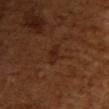<lesion>
  <biopsy_status>not biopsied; imaged during a skin examination</biopsy_status>
  <automated_metrics>
    <cielab_L>24</cielab_L>
    <cielab_a>20</cielab_a>
    <cielab_b>28</cielab_b>
    <vs_skin_darker_L>5.0</vs_skin_darker_L>
    <vs_skin_contrast_norm>6.0</vs_skin_contrast_norm>
    <color_variation_0_10>1.5</color_variation_0_10>
    <peripheral_color_asymmetry>0.5</peripheral_color_asymmetry>
  </automated_metrics>
  <site>upper back</site>
  <lesion_size>
    <long_diameter_mm_approx>2.5</long_diameter_mm_approx>
  </lesion_size>
  <image>
    <source>total-body photography crop</source>
    <field_of_view_mm>15</field_of_view_mm>
  </image>
  <lighting>cross-polarized</lighting>
  <patient>
    <sex>female</sex>
    <age_approx>55</age_approx>
  </patient>
</lesion>